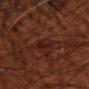Imaged during a routine full-body skin examination; the lesion was not biopsied and no histopathology is available. The lesion is located on the right upper arm. About 2.5 mm across. A 15 mm close-up tile from a total-body photography series done for melanoma screening. The patient is a male roughly 70 years of age.